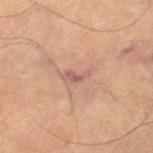size: ≈2.5 mm
tile lighting: cross-polarized
image-analysis metrics: an area of roughly 2.5 mm², an outline eccentricity of about 0.9 (0 = round, 1 = elongated), and two-axis asymmetry of about 0.5; an average lesion color of about L≈45 a*≈20 b*≈21 (CIELAB), roughly 7 lightness units darker than nearby skin, and a lesion-to-skin contrast of about 6.5 (normalized; higher = more distinct); a border-irregularity rating of about 5.5/10 and radial color variation of about 0
site: the right thigh
subject: female, in their 60s
acquisition: ~15 mm tile from a whole-body skin photo